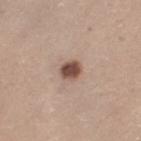Q: Was this lesion biopsied?
A: total-body-photography surveillance lesion; no biopsy
Q: Patient demographics?
A: female, aged approximately 45
Q: What kind of image is this?
A: ~15 mm tile from a whole-body skin photo
Q: What is the anatomic site?
A: the left thigh